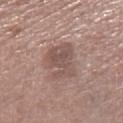Q: Was this lesion biopsied?
A: no biopsy performed (imaged during a skin exam)
Q: What is the anatomic site?
A: the leg
Q: What is the imaging modality?
A: total-body-photography crop, ~15 mm field of view
Q: Who is the patient?
A: female, roughly 75 years of age